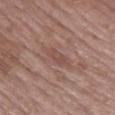The lesion is located on the right forearm.
A male subject in their 70s.
Measured at roughly 3.5 mm in maximum diameter.
This is a white-light tile.
Automated image analysis of the tile measured a lesion area of about 5 mm² and an eccentricity of roughly 0.9. And it measured a border-irregularity index near 3/10, a within-lesion color-variation index near 2/10, and radial color variation of about 1.
Cropped from a total-body skin-imaging series; the visible field is about 15 mm.